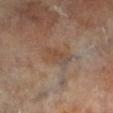{
  "lesion_size": {
    "long_diameter_mm_approx": 4.0
  },
  "automated_metrics": {
    "area_mm2_approx": 7.0,
    "shape_asymmetry": 0.2,
    "nevus_likeness_0_100": 0
  },
  "image": {
    "source": "total-body photography crop",
    "field_of_view_mm": 15
  },
  "site": "left lower leg",
  "patient": {
    "sex": "male",
    "age_approx": 65
  }
}